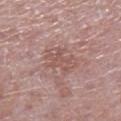| key | value |
|---|---|
| notes | catalogued during a skin exam; not biopsied |
| image source | ~15 mm tile from a whole-body skin photo |
| body site | the left lower leg |
| tile lighting | white-light illumination |
| subject | male, aged 73 to 77 |